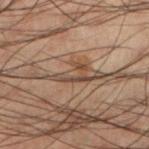The lesion was tiled from a total-body skin photograph and was not biopsied. Imaged with cross-polarized lighting. Cropped from a whole-body photographic skin survey; the tile spans about 15 mm. Longest diameter approximately 3.5 mm. Located on the left lower leg. A male subject aged 48 to 52. Automated image analysis of the tile measured a footprint of about 5 mm², an outline eccentricity of about 0.8 (0 = round, 1 = elongated), and a symmetry-axis asymmetry near 0.5. And it measured a normalized lesion–skin contrast near 7. The analysis additionally found a nevus-likeness score of about 0/100 and a detector confidence of about 65 out of 100 that the crop contains a lesion.